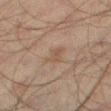biopsy status — catalogued during a skin exam; not biopsied
subject — male, aged around 50
TBP lesion metrics — an area of roughly 3.5 mm², a shape eccentricity near 0.75, and a shape-asymmetry score of about 0.4 (0 = symmetric); a border-irregularity index near 4/10, a color-variation rating of about 1.5/10, and radial color variation of about 0.5; a detector confidence of about 100 out of 100 that the crop contains a lesion
anatomic site — the leg
image — 15 mm crop, total-body photography
tile lighting — cross-polarized
diameter — about 2.5 mm